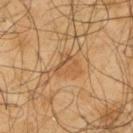<tbp_lesion>
<biopsy_status>not biopsied; imaged during a skin examination</biopsy_status>
<image>
  <source>total-body photography crop</source>
  <field_of_view_mm>15</field_of_view_mm>
</image>
<lesion_size>
  <long_diameter_mm_approx>4.0</long_diameter_mm_approx>
</lesion_size>
<automated_metrics>
  <lesion_detection_confidence_0_100>80</lesion_detection_confidence_0_100>
</automated_metrics>
<site>back</site>
<patient>
  <sex>male</sex>
  <age_approx>65</age_approx>
</patient>
<lighting>cross-polarized</lighting>
</tbp_lesion>